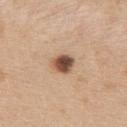workup: catalogued during a skin exam; not biopsied
patient: female, in their mid- to late 40s
image: 15 mm crop, total-body photography
anatomic site: the upper back
tile lighting: white-light
size: about 2.5 mm